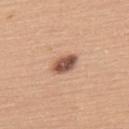Imaged during a routine full-body skin examination; the lesion was not biopsied and no histopathology is available.
On the upper back.
A female patient aged 63 to 67.
Cropped from a total-body skin-imaging series; the visible field is about 15 mm.
This is a white-light tile.
About 3.5 mm across.